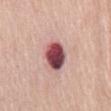notes = catalogued during a skin exam; not biopsied
illumination = white-light illumination
patient = female, in their mid- to late 60s
location = the mid back
image source = ~15 mm tile from a whole-body skin photo
size = about 4 mm